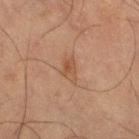The lesion was tiled from a total-body skin photograph and was not biopsied. A male patient aged approximately 65. A lesion tile, about 15 mm wide, cut from a 3D total-body photograph. From the left thigh.